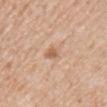Recorded during total-body skin imaging; not selected for excision or biopsy.
The subject is a female aged 68–72.
Located on the chest.
The total-body-photography lesion software estimated a lesion area of about 4.5 mm² and an outline eccentricity of about 0.7 (0 = round, 1 = elongated). And it measured a mean CIELAB color near L≈63 a*≈19 b*≈33, about 8 CIELAB-L* units darker than the surrounding skin, and a normalized lesion–skin contrast near 5.5. The software also gave a classifier nevus-likeness of about 5/100 and a detector confidence of about 100 out of 100 that the crop contains a lesion.
A 15 mm crop from a total-body photograph taken for skin-cancer surveillance.